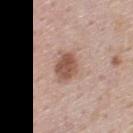{
  "site": "upper back",
  "lighting": "white-light",
  "lesion_size": {
    "long_diameter_mm_approx": 3.5
  },
  "patient": {
    "sex": "male",
    "age_approx": 45
  },
  "automated_metrics": {
    "eccentricity": 0.6,
    "shape_asymmetry": 0.2,
    "cielab_L": 54,
    "cielab_a": 20,
    "cielab_b": 27,
    "vs_skin_darker_L": 12.0,
    "vs_skin_contrast_norm": 8.5,
    "border_irregularity_0_10": 2.0,
    "color_variation_0_10": 3.0,
    "nevus_likeness_0_100": 90,
    "lesion_detection_confidence_0_100": 100
  },
  "image": {
    "source": "total-body photography crop",
    "field_of_view_mm": 15
  }
}